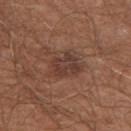{
  "site": "right upper arm",
  "image": {
    "source": "total-body photography crop",
    "field_of_view_mm": 15
  },
  "patient": {
    "sex": "male",
    "age_approx": 65
  }
}